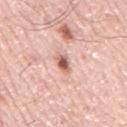biopsy_status: not biopsied; imaged during a skin examination
patient:
  sex: male
  age_approx: 75
automated_metrics:
  area_mm2_approx: 3.5
  border_irregularity_0_10: 2.0
  color_variation_0_10: 4.0
  peripheral_color_asymmetry: 1.0
  nevus_likeness_0_100: 100
  lesion_detection_confidence_0_100: 100
lighting: white-light
lesion_size:
  long_diameter_mm_approx: 2.5
site: mid back
image:
  source: total-body photography crop
  field_of_view_mm: 15